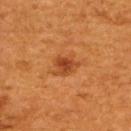Recorded during total-body skin imaging; not selected for excision or biopsy.
Cropped from a whole-body photographic skin survey; the tile spans about 15 mm.
An algorithmic analysis of the crop reported a lesion area of about 6.5 mm² and two-axis asymmetry of about 0.3. The analysis additionally found a lesion color around L≈38 a*≈25 b*≈36 in CIELAB, roughly 8 lightness units darker than nearby skin, and a lesion-to-skin contrast of about 7 (normalized; higher = more distinct).
The lesion is located on the upper back.
This is a cross-polarized tile.
About 3.5 mm across.
A female patient, aged around 50.